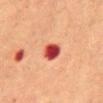Q: Was this lesion biopsied?
A: total-body-photography surveillance lesion; no biopsy
Q: What kind of image is this?
A: ~15 mm crop, total-body skin-cancer survey
Q: Where on the body is the lesion?
A: the front of the torso
Q: Who is the patient?
A: female, aged 68–72